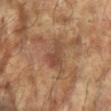notes: imaged on a skin check; not biopsied | anatomic site: the right arm | image: ~15 mm tile from a whole-body skin photo | subject: female, approximately 80 years of age | lighting: cross-polarized illumination | lesion diameter: ≈3.5 mm | TBP lesion metrics: a lesion area of about 6 mm², an outline eccentricity of about 0.8 (0 = round, 1 = elongated), and a shape-asymmetry score of about 0.5 (0 = symmetric); border irregularity of about 5 on a 0–10 scale, internal color variation of about 3 on a 0–10 scale, and peripheral color asymmetry of about 1.